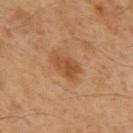This lesion was catalogued during total-body skin photography and was not selected for biopsy. The lesion is on the back. The patient is a male about 60 years old. A roughly 15 mm field-of-view crop from a total-body skin photograph. The total-body-photography lesion software estimated an area of roughly 7.5 mm² and two-axis asymmetry of about 0.25. And it measured a mean CIELAB color near L≈45 a*≈21 b*≈34, about 8 CIELAB-L* units darker than the surrounding skin, and a normalized lesion–skin contrast near 7. And it measured a border-irregularity rating of about 2.5/10 and a color-variation rating of about 3/10. This is a cross-polarized tile. Approximately 4 mm at its widest.